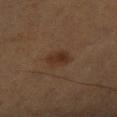No biopsy was performed on this lesion — it was imaged during a full skin examination and was not determined to be concerning. A roughly 15 mm field-of-view crop from a total-body skin photograph. The patient is a male approximately 55 years of age. The lesion is located on the leg.